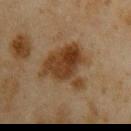Clinical impression: This lesion was catalogued during total-body skin photography and was not selected for biopsy. Image and clinical context: From the right upper arm. About 6 mm across. Automated tile analysis of the lesion measured a mean CIELAB color near L≈31 a*≈15 b*≈27 and a normalized lesion–skin contrast near 10. And it measured a border-irregularity rating of about 5/10, a within-lesion color-variation index near 5/10, and radial color variation of about 2. And it measured a classifier nevus-likeness of about 85/100 and lesion-presence confidence of about 100/100. Cropped from a total-body skin-imaging series; the visible field is about 15 mm. This is a cross-polarized tile. A male patient aged approximately 45.